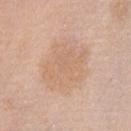{
  "biopsy_status": "not biopsied; imaged during a skin examination",
  "patient": {
    "sex": "female",
    "age_approx": 65
  },
  "image": {
    "source": "total-body photography crop",
    "field_of_view_mm": 15
  },
  "lighting": "white-light",
  "site": "abdomen",
  "lesion_size": {
    "long_diameter_mm_approx": 7.0
  }
}